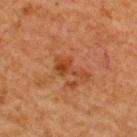Notes:
- follow-up — catalogued during a skin exam; not biopsied
- location — the upper back
- size — ~4 mm (longest diameter)
- illumination — cross-polarized
- subject — male, roughly 65 years of age
- TBP lesion metrics — a lesion area of about 5 mm² and an eccentricity of roughly 0.9; a lesion color around L≈39 a*≈25 b*≈35 in CIELAB, about 8 CIELAB-L* units darker than the surrounding skin, and a lesion-to-skin contrast of about 7 (normalized; higher = more distinct); an automated nevus-likeness rating near 0 out of 100 and lesion-presence confidence of about 100/100
- imaging modality — ~15 mm crop, total-body skin-cancer survey